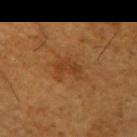Q: Was this lesion biopsied?
A: catalogued during a skin exam; not biopsied
Q: What is the imaging modality?
A: total-body-photography crop, ~15 mm field of view
Q: What is the lesion's diameter?
A: about 3 mm
Q: Who is the patient?
A: male, roughly 65 years of age
Q: What is the anatomic site?
A: the right upper arm